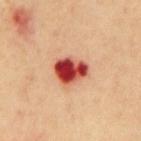biopsy status: imaged on a skin check; not biopsied
location: the abdomen
automated lesion analysis: a lesion area of about 10 mm², an eccentricity of roughly 0.65, and a shape-asymmetry score of about 0.2 (0 = symmetric)
subject: male, aged approximately 70
imaging modality: ~15 mm tile from a whole-body skin photo
lighting: cross-polarized illumination
lesion diameter: ~4 mm (longest diameter)